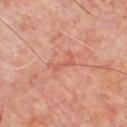Part of a total-body skin-imaging series; this lesion was reviewed on a skin check and was not flagged for biopsy. The lesion is located on the chest. A 15 mm close-up extracted from a 3D total-body photography capture. The patient is a male approximately 65 years of age. Approximately 3.5 mm at its widest. Imaged with cross-polarized lighting.